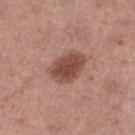biopsy status: imaged on a skin check; not biopsied | image source: total-body-photography crop, ~15 mm field of view | patient: female, aged around 40 | anatomic site: the right thigh.